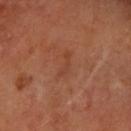Q: Is there a histopathology result?
A: imaged on a skin check; not biopsied
Q: Lesion location?
A: the left forearm
Q: Patient demographics?
A: female, aged approximately 60
Q: How was this image acquired?
A: ~15 mm tile from a whole-body skin photo